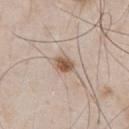The lesion was tiled from a total-body skin photograph and was not biopsied.
The subject is a male about 55 years old.
A 15 mm close-up tile from a total-body photography series done for melanoma screening.
Imaged with white-light lighting.
Located on the front of the torso.
Approximately 2.5 mm at its widest.